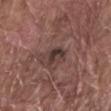biopsy status: total-body-photography surveillance lesion; no biopsy